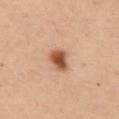Imaged during a routine full-body skin examination; the lesion was not biopsied and no histopathology is available. A female patient, in their mid- to late 50s. Imaged with cross-polarized lighting. The lesion is on the upper back. The total-body-photography lesion software estimated a mean CIELAB color near L≈44 a*≈20 b*≈29 and a normalized lesion–skin contrast near 10.5. It also reported border irregularity of about 3 on a 0–10 scale and a within-lesion color-variation index near 3.5/10. Cropped from a whole-body photographic skin survey; the tile spans about 15 mm. Measured at roughly 3.5 mm in maximum diameter.